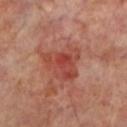{
  "image": {
    "source": "total-body photography crop",
    "field_of_view_mm": 15
  },
  "automated_metrics": {
    "area_mm2_approx": 12.0,
    "eccentricity": 0.7,
    "shape_asymmetry": 0.45
  },
  "lighting": "cross-polarized",
  "patient": {
    "sex": "male",
    "age_approx": 70
  },
  "site": "leg"
}